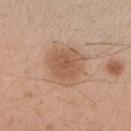Clinical impression: Part of a total-body skin-imaging series; this lesion was reviewed on a skin check and was not flagged for biopsy. Context: The total-body-photography lesion software estimated an average lesion color of about L≈57 a*≈20 b*≈31 (CIELAB), roughly 9 lightness units darker than nearby skin, and a normalized lesion–skin contrast near 6.5. Longest diameter approximately 4.5 mm. This image is a 15 mm lesion crop taken from a total-body photograph. A female subject, approximately 40 years of age. Located on the right forearm. This is a white-light tile.